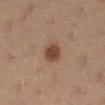{
  "biopsy_status": "not biopsied; imaged during a skin examination",
  "lesion_size": {
    "long_diameter_mm_approx": 2.5
  },
  "image": {
    "source": "total-body photography crop",
    "field_of_view_mm": 15
  },
  "automated_metrics": {
    "vs_skin_darker_L": 11.0,
    "vs_skin_contrast_norm": 8.5
  },
  "site": "leg",
  "patient": {
    "sex": "female",
    "age_approx": 35
  },
  "lighting": "cross-polarized"
}